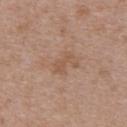follow-up = no biopsy performed (imaged during a skin exam) | location = the upper back | imaging modality = ~15 mm tile from a whole-body skin photo | subject = female, aged 28 to 32.